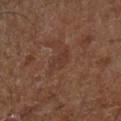From the leg. This is a cross-polarized tile. Automated tile analysis of the lesion measured an area of roughly 4 mm², a shape eccentricity near 0.8, and a symmetry-axis asymmetry near 0.4. It also reported a normalized lesion–skin contrast near 4.5. It also reported border irregularity of about 4 on a 0–10 scale, a within-lesion color-variation index near 2.5/10, and radial color variation of about 1. The subject is roughly 65 years of age. A lesion tile, about 15 mm wide, cut from a 3D total-body photograph.